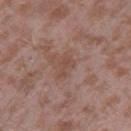* notes · total-body-photography surveillance lesion; no biopsy
* lesion diameter · ~3 mm (longest diameter)
* site · the right thigh
* lighting · white-light
* patient · male, in their mid-40s
* image · 15 mm crop, total-body photography